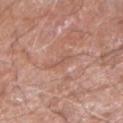Assessment:
No biopsy was performed on this lesion — it was imaged during a full skin examination and was not determined to be concerning.
Clinical summary:
The tile uses white-light illumination. A close-up tile cropped from a whole-body skin photograph, about 15 mm across. A male subject aged 58 to 62. Approximately 2.5 mm at its widest. The lesion is located on the right forearm. An algorithmic analysis of the crop reported a mean CIELAB color near L≈55 a*≈22 b*≈28 and about 6 CIELAB-L* units darker than the surrounding skin. And it measured a border-irregularity rating of about 6/10 and peripheral color asymmetry of about 0.